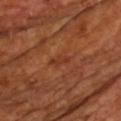biopsy_status: not biopsied; imaged during a skin examination
lighting: cross-polarized
lesion_size:
  long_diameter_mm_approx: 2.5
image:
  source: total-body photography crop
  field_of_view_mm: 15
patient:
  sex: male
  age_approx: 65